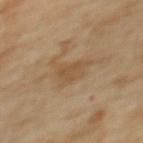  biopsy_status: not biopsied; imaged during a skin examination
  patient:
    sex: female
    age_approx: 60
  lesion_size:
    long_diameter_mm_approx: 3.5
  image:
    source: total-body photography crop
    field_of_view_mm: 15
  site: upper back